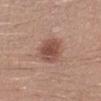follow-up: catalogued during a skin exam; not biopsied
patient: male, roughly 30 years of age
location: the leg
TBP lesion metrics: a lesion color around L≈49 a*≈22 b*≈25 in CIELAB, roughly 12 lightness units darker than nearby skin, and a lesion-to-skin contrast of about 8.5 (normalized; higher = more distinct); a color-variation rating of about 3/10; lesion-presence confidence of about 100/100
acquisition: total-body-photography crop, ~15 mm field of view
illumination: white-light illumination
diameter: ~4 mm (longest diameter)